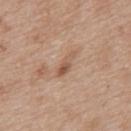No biopsy was performed on this lesion — it was imaged during a full skin examination and was not determined to be concerning.
A 15 mm crop from a total-body photograph taken for skin-cancer surveillance.
A female subject aged around 40.
The lesion's longest dimension is about 4 mm.
The lesion is located on the upper back.